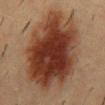– notes: total-body-photography surveillance lesion; no biopsy
– location: the mid back
– acquisition: ~15 mm crop, total-body skin-cancer survey
– lighting: cross-polarized illumination
– diameter: about 11.5 mm
– subject: male, roughly 55 years of age